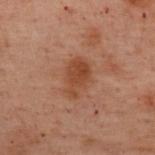The lesion was photographed on a routine skin check and not biopsied; there is no pathology result. The subject is a female approximately 55 years of age. A 15 mm close-up extracted from a 3D total-body photography capture. The lesion is located on the upper back. The recorded lesion diameter is about 4 mm. Automated image analysis of the tile measured a border-irregularity rating of about 3/10, a color-variation rating of about 2.5/10, and radial color variation of about 1.